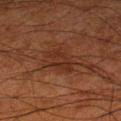The lesion was photographed on a routine skin check and not biopsied; there is no pathology result.
Automated image analysis of the tile measured an average lesion color of about L≈23 a*≈19 b*≈23 (CIELAB), about 5 CIELAB-L* units darker than the surrounding skin, and a normalized border contrast of about 6.
The lesion is located on the left lower leg.
A close-up tile cropped from a whole-body skin photograph, about 15 mm across.
Captured under cross-polarized illumination.
About 3.5 mm across.
A male subject aged 78–82.